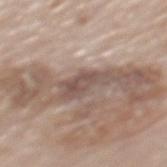Q: Where on the body is the lesion?
A: the mid back
Q: How large is the lesion?
A: ≈5 mm
Q: What are the patient's age and sex?
A: male, aged 73–77
Q: How was this image acquired?
A: total-body-photography crop, ~15 mm field of view
Q: Illumination type?
A: white-light